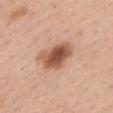Part of a total-body skin-imaging series; this lesion was reviewed on a skin check and was not flagged for biopsy. Automated tile analysis of the lesion measured a lesion color around L≈55 a*≈23 b*≈31 in CIELAB. And it measured a border-irregularity rating of about 2.5/10, a color-variation rating of about 6/10, and peripheral color asymmetry of about 2. The recorded lesion diameter is about 5 mm. A close-up tile cropped from a whole-body skin photograph, about 15 mm across. From the upper back. Imaged with white-light lighting. A female patient aged approximately 35.